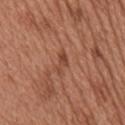Q: Was this lesion biopsied?
A: total-body-photography surveillance lesion; no biopsy
Q: Lesion location?
A: the mid back
Q: What did automated image analysis measure?
A: an average lesion color of about L≈45 a*≈24 b*≈31 (CIELAB), about 8 CIELAB-L* units darker than the surrounding skin, and a lesion-to-skin contrast of about 6.5 (normalized; higher = more distinct); border irregularity of about 6.5 on a 0–10 scale and a within-lesion color-variation index near 0/10; an automated nevus-likeness rating near 0 out of 100 and a lesion-detection confidence of about 100/100
Q: How was the tile lit?
A: white-light
Q: How was this image acquired?
A: ~15 mm tile from a whole-body skin photo
Q: Who is the patient?
A: male, aged around 65